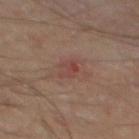patient = male, in their mid-60s
image-analysis metrics = a footprint of about 3.5 mm², an eccentricity of roughly 0.85, and a symmetry-axis asymmetry near 0.3; border irregularity of about 4 on a 0–10 scale, a color-variation rating of about 3/10, and peripheral color asymmetry of about 1
illumination = cross-polarized
imaging modality = total-body-photography crop, ~15 mm field of view
body site = the back